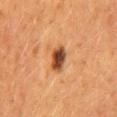workup = total-body-photography surveillance lesion; no biopsy
tile lighting = cross-polarized illumination
body site = the back
lesion size = ≈3.5 mm
patient = female, roughly 50 years of age
automated lesion analysis = a mean CIELAB color near L≈38 a*≈22 b*≈32 and a normalized lesion–skin contrast near 12.5
imaging modality = ~15 mm tile from a whole-body skin photo